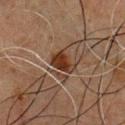Impression: Part of a total-body skin-imaging series; this lesion was reviewed on a skin check and was not flagged for biopsy. Acquisition and patient details: The lesion's longest dimension is about 3 mm. Imaged with cross-polarized lighting. A lesion tile, about 15 mm wide, cut from a 3D total-body photograph. From the chest. A male patient about 50 years old.